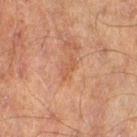{"biopsy_status": "not biopsied; imaged during a skin examination", "site": "right thigh", "lesion_size": {"long_diameter_mm_approx": 2.5}, "patient": {"sex": "male", "age_approx": 70}, "image": {"source": "total-body photography crop", "field_of_view_mm": 15}, "automated_metrics": {"area_mm2_approx": 2.0, "eccentricity": 0.9, "cielab_L": 45, "cielab_a": 21, "cielab_b": 29, "vs_skin_darker_L": 6.0, "vs_skin_contrast_norm": 5.0, "color_variation_0_10": 0.0, "nevus_likeness_0_100": 0, "lesion_detection_confidence_0_100": 100}}